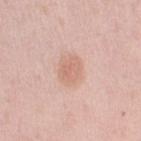{"biopsy_status": "not biopsied; imaged during a skin examination", "lighting": "white-light", "image": {"source": "total-body photography crop", "field_of_view_mm": 15}, "site": "right upper arm", "lesion_size": {"long_diameter_mm_approx": 3.5}, "patient": {"sex": "female", "age_approx": 40}}